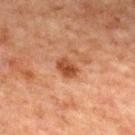Clinical impression: Part of a total-body skin-imaging series; this lesion was reviewed on a skin check and was not flagged for biopsy. Background: The total-body-photography lesion software estimated an area of roughly 4.5 mm², an eccentricity of roughly 0.7, and a symmetry-axis asymmetry near 0.2. It also reported a lesion color around L≈39 a*≈23 b*≈31 in CIELAB, a lesion–skin lightness drop of about 10, and a lesion-to-skin contrast of about 8.5 (normalized; higher = more distinct). Imaged with cross-polarized lighting. Cropped from a whole-body photographic skin survey; the tile spans about 15 mm. A male subject aged 63–67. The lesion is located on the chest. The recorded lesion diameter is about 3 mm.